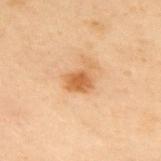No biopsy was performed on this lesion — it was imaged during a full skin examination and was not determined to be concerning. Cropped from a total-body skin-imaging series; the visible field is about 15 mm. A male patient aged approximately 55. The lesion's longest dimension is about 3 mm. Located on the upper back. The tile uses cross-polarized illumination.